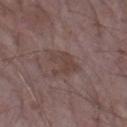Recorded during total-body skin imaging; not selected for excision or biopsy. A male subject, roughly 65 years of age. An algorithmic analysis of the crop reported an outline eccentricity of about 0.7 (0 = round, 1 = elongated) and a shape-asymmetry score of about 0.45 (0 = symmetric). The software also gave roughly 6 lightness units darker than nearby skin and a normalized border contrast of about 6. It also reported a nevus-likeness score of about 0/100 and a lesion-detection confidence of about 100/100. Captured under white-light illumination. A lesion tile, about 15 mm wide, cut from a 3D total-body photograph. From the left forearm. Longest diameter approximately 4 mm.